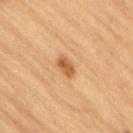The lesion was photographed on a routine skin check and not biopsied; there is no pathology result.
From the lower back.
A male subject aged around 85 or older.
Automated image analysis of the tile measured a footprint of about 4 mm², an eccentricity of roughly 0.8, and a symmetry-axis asymmetry near 0.2. The software also gave internal color variation of about 3 on a 0–10 scale and radial color variation of about 1.
This image is a 15 mm lesion crop taken from a total-body photograph.
The lesion's longest dimension is about 2.5 mm.
The tile uses cross-polarized illumination.